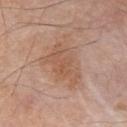The lesion was tiled from a total-body skin photograph and was not biopsied. Longest diameter approximately 5.5 mm. The total-body-photography lesion software estimated an area of roughly 17 mm². It also reported roughly 7 lightness units darker than nearby skin. From the chest. A 15 mm close-up extracted from a 3D total-body photography capture. Imaged with white-light lighting. A male patient in their mid- to late 50s.